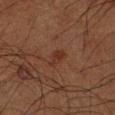Located on the arm.
A male subject, roughly 60 years of age.
A region of skin cropped from a whole-body photographic capture, roughly 15 mm wide.
Approximately 2.5 mm at its widest.
This is a cross-polarized tile.